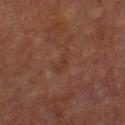Acquisition and patient details:
The tile uses cross-polarized illumination. Located on the upper back. A 15 mm close-up tile from a total-body photography series done for melanoma screening. The total-body-photography lesion software estimated an area of roughly 3 mm², an eccentricity of roughly 0.95, and a shape-asymmetry score of about 0.45 (0 = symmetric). The software also gave radial color variation of about 0. The analysis additionally found a lesion-detection confidence of about 100/100. The subject is a male approximately 65 years of age.